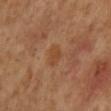This lesion was catalogued during total-body skin photography and was not selected for biopsy. The subject is a male aged around 60. The lesion-visualizer software estimated an area of roughly 4.5 mm² and a shape eccentricity near 0.65. The software also gave an average lesion color of about L≈41 a*≈19 b*≈32 (CIELAB) and a normalized border contrast of about 5.5. It also reported border irregularity of about 2.5 on a 0–10 scale, a within-lesion color-variation index near 1.5/10, and peripheral color asymmetry of about 0.5. The software also gave a nevus-likeness score of about 0/100. A roughly 15 mm field-of-view crop from a total-body skin photograph. The lesion is located on the mid back. Imaged with cross-polarized lighting.